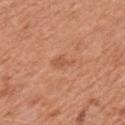Captured under white-light illumination.
A male patient, about 70 years old.
An algorithmic analysis of the crop reported border irregularity of about 4 on a 0–10 scale, a within-lesion color-variation index near 1/10, and a peripheral color-asymmetry measure near 0.5.
The lesion is located on the left upper arm.
A 15 mm crop from a total-body photograph taken for skin-cancer surveillance.
The recorded lesion diameter is about 3 mm.